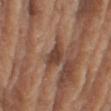The lesion was photographed on a routine skin check and not biopsied; there is no pathology result. A male patient approximately 75 years of age. Automated image analysis of the tile measured a mean CIELAB color near L≈43 a*≈19 b*≈27 and about 10 CIELAB-L* units darker than the surrounding skin. It also reported a peripheral color-asymmetry measure near 1. The software also gave a classifier nevus-likeness of about 0/100 and a detector confidence of about 100 out of 100 that the crop contains a lesion. The recorded lesion diameter is about 4 mm. The lesion is located on the right upper arm. A 15 mm crop from a total-body photograph taken for skin-cancer surveillance.